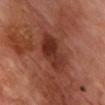follow-up = total-body-photography surveillance lesion; no biopsy | subject = male, about 70 years old | illumination = cross-polarized illumination | image source = 15 mm crop, total-body photography | size = ~5 mm (longest diameter) | anatomic site = the chest.